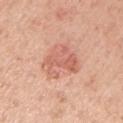<case>
  <biopsy_status>not biopsied; imaged during a skin examination</biopsy_status>
  <lighting>white-light</lighting>
  <lesion_size>
    <long_diameter_mm_approx>5.0</long_diameter_mm_approx>
  </lesion_size>
  <image>
    <source>total-body photography crop</source>
    <field_of_view_mm>15</field_of_view_mm>
  </image>
  <site>left upper arm</site>
  <patient>
    <sex>male</sex>
    <age_approx>75</age_approx>
  </patient>
</case>